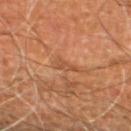The lesion was photographed on a routine skin check and not biopsied; there is no pathology result.
On the leg.
An algorithmic analysis of the crop reported a footprint of about 2.5 mm², an outline eccentricity of about 0.85 (0 = round, 1 = elongated), and a symmetry-axis asymmetry near 0.45. And it measured a lesion–skin lightness drop of about 7. It also reported a nevus-likeness score of about 0/100 and lesion-presence confidence of about 70/100.
This is a cross-polarized tile.
A male patient, aged approximately 60.
About 2.5 mm across.
A 15 mm crop from a total-body photograph taken for skin-cancer surveillance.